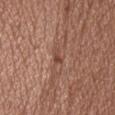Assessment: The lesion was tiled from a total-body skin photograph and was not biopsied. Background: The lesion is located on the head or neck. Approximately 2.5 mm at its widest. A male subject about 35 years old. A roughly 15 mm field-of-view crop from a total-body skin photograph. The tile uses white-light illumination.